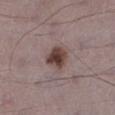Automated tile analysis of the lesion measured a footprint of about 6.5 mm² and a shape-asymmetry score of about 0.25 (0 = symmetric). The software also gave border irregularity of about 2 on a 0–10 scale, a within-lesion color-variation index near 4/10, and radial color variation of about 1.5.
On the left lower leg.
Measured at roughly 3 mm in maximum diameter.
A male patient about 70 years old.
A close-up tile cropped from a whole-body skin photograph, about 15 mm across.
Captured under white-light illumination.